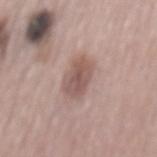The lesion was tiled from a total-body skin photograph and was not biopsied.
The tile uses white-light illumination.
Cropped from a whole-body photographic skin survey; the tile spans about 15 mm.
The lesion-visualizer software estimated an area of roughly 9 mm² and a symmetry-axis asymmetry near 0.2. And it measured a lesion–skin lightness drop of about 11.
The subject is a male aged approximately 55.